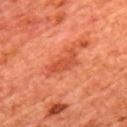This lesion was catalogued during total-body skin photography and was not selected for biopsy.